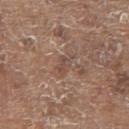* notes: imaged on a skin check; not biopsied
* automated lesion analysis: a lesion area of about 3.5 mm² and two-axis asymmetry of about 0.4; a classifier nevus-likeness of about 0/100 and lesion-presence confidence of about 75/100
* lighting: white-light illumination
* patient: male, in their 80s
* lesion size: ~3 mm (longest diameter)
* body site: the back
* image: total-body-photography crop, ~15 mm field of view